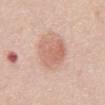Clinical impression: Imaged during a routine full-body skin examination; the lesion was not biopsied and no histopathology is available. Background: The subject is a male aged approximately 50. A close-up tile cropped from a whole-body skin photograph, about 15 mm across. From the abdomen.